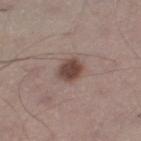No biopsy was performed on this lesion — it was imaged during a full skin examination and was not determined to be concerning. A male patient roughly 60 years of age. This is a white-light tile. Automated image analysis of the tile measured an outline eccentricity of about 0.7 (0 = round, 1 = elongated) and a shape-asymmetry score of about 0.2 (0 = symmetric). It also reported a nevus-likeness score of about 95/100 and lesion-presence confidence of about 100/100. Located on the left thigh. Cropped from a whole-body photographic skin survey; the tile spans about 15 mm. Longest diameter approximately 3.5 mm.